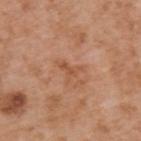notes: catalogued during a skin exam; not biopsied
illumination: white-light illumination
body site: the upper back
subject: male, in their mid- to late 60s
image source: 15 mm crop, total-body photography